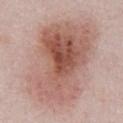The lesion was photographed on a routine skin check and not biopsied; there is no pathology result. The lesion is on the abdomen. Imaged with white-light lighting. A female patient roughly 50 years of age. Cropped from a total-body skin-imaging series; the visible field is about 15 mm.